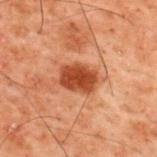The lesion was tiled from a total-body skin photograph and was not biopsied.
The lesion is located on the upper back.
Captured under cross-polarized illumination.
A 15 mm crop from a total-body photograph taken for skin-cancer surveillance.
The patient is a male in their 60s.